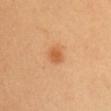biopsy status: imaged on a skin check; not biopsied
image: ~15 mm tile from a whole-body skin photo
illumination: cross-polarized illumination
size: ≈2.5 mm
patient: female, aged 38 to 42
site: the head or neck